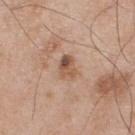Clinical impression:
Part of a total-body skin-imaging series; this lesion was reviewed on a skin check and was not flagged for biopsy.